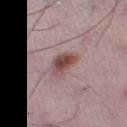Recorded during total-body skin imaging; not selected for excision or biopsy.
A roughly 15 mm field-of-view crop from a total-body skin photograph.
The lesion is on the right thigh.
The recorded lesion diameter is about 3.5 mm.
An algorithmic analysis of the crop reported a lesion color around L≈48 a*≈20 b*≈20 in CIELAB, roughly 13 lightness units darker than nearby skin, and a normalized lesion–skin contrast near 9.5. And it measured a border-irregularity index near 3/10, a within-lesion color-variation index near 4.5/10, and peripheral color asymmetry of about 1.5.
The patient is a male aged 48–52.
Captured under white-light illumination.